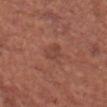The lesion was photographed on a routine skin check and not biopsied; there is no pathology result.
The subject is a male approximately 65 years of age.
The lesion's longest dimension is about 3 mm.
From the head or neck.
This is a white-light tile.
A 15 mm close-up tile from a total-body photography series done for melanoma screening.